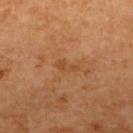| key | value |
|---|---|
| workup | no biopsy performed (imaged during a skin exam) |
| automated lesion analysis | a footprint of about 3 mm², an outline eccentricity of about 0.9 (0 = round, 1 = elongated), and a shape-asymmetry score of about 0.4 (0 = symmetric); internal color variation of about 0 on a 0–10 scale and peripheral color asymmetry of about 0 |
| diameter | about 2.5 mm |
| lighting | cross-polarized |
| imaging modality | ~15 mm crop, total-body skin-cancer survey |
| patient | male, approximately 60 years of age |
| location | the upper back |